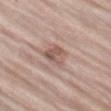Clinical impression:
This lesion was catalogued during total-body skin photography and was not selected for biopsy.
Acquisition and patient details:
A male patient, aged around 80. Approximately 3 mm at its widest. A 15 mm close-up extracted from a 3D total-body photography capture. Imaged with white-light lighting. An algorithmic analysis of the crop reported a border-irregularity rating of about 3.5/10 and a color-variation rating of about 4/10. The software also gave a classifier nevus-likeness of about 0/100 and a lesion-detection confidence of about 100/100. On the left thigh.